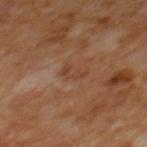Clinical impression: Captured during whole-body skin photography for melanoma surveillance; the lesion was not biopsied. Image and clinical context: The total-body-photography lesion software estimated a footprint of about 2.5 mm², an outline eccentricity of about 0.9 (0 = round, 1 = elongated), and a symmetry-axis asymmetry near 0.55. The analysis additionally found a lesion color around L≈39 a*≈19 b*≈30 in CIELAB, a lesion–skin lightness drop of about 5, and a normalized lesion–skin contrast near 5. The analysis additionally found a border-irregularity rating of about 6.5/10, a within-lesion color-variation index near 0/10, and peripheral color asymmetry of about 0. It also reported a classifier nevus-likeness of about 0/100 and a lesion-detection confidence of about 100/100. The recorded lesion diameter is about 2.5 mm. A 15 mm crop from a total-body photograph taken for skin-cancer surveillance. Captured under cross-polarized illumination. From the mid back. A male patient, aged around 65.